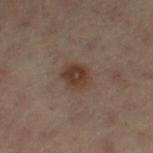Part of a total-body skin-imaging series; this lesion was reviewed on a skin check and was not flagged for biopsy.
A 15 mm crop from a total-body photograph taken for skin-cancer surveillance.
From the left thigh.
The recorded lesion diameter is about 3 mm.
The patient is a female aged around 55.
Automated tile analysis of the lesion measured a nevus-likeness score of about 90/100 and a detector confidence of about 100 out of 100 that the crop contains a lesion.
The tile uses cross-polarized illumination.